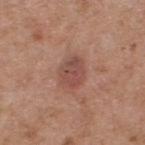The lesion was photographed on a routine skin check and not biopsied; there is no pathology result. Automated tile analysis of the lesion measured a footprint of about 7.5 mm², an eccentricity of roughly 0.65, and a symmetry-axis asymmetry near 0.2. It also reported a border-irregularity rating of about 2/10, a color-variation rating of about 4.5/10, and radial color variation of about 1.5. A male patient about 55 years old. The lesion's longest dimension is about 3.5 mm. A 15 mm close-up extracted from a 3D total-body photography capture. This is a white-light tile. Located on the back.